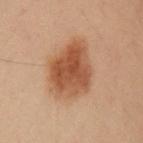Case summary:
– notes: imaged on a skin check; not biopsied
– image source: ~15 mm tile from a whole-body skin photo
– illumination: cross-polarized
– patient: male, aged 53 to 57
– anatomic site: the left upper arm
– automated lesion analysis: an average lesion color of about L≈42 a*≈19 b*≈29 (CIELAB), about 11 CIELAB-L* units darker than the surrounding skin, and a normalized border contrast of about 9; a color-variation rating of about 4.5/10 and a peripheral color-asymmetry measure near 1.5; a nevus-likeness score of about 100/100 and lesion-presence confidence of about 100/100